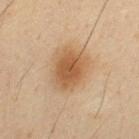The lesion was photographed on a routine skin check and not biopsied; there is no pathology result. A 15 mm crop from a total-body photograph taken for skin-cancer surveillance. A male subject aged approximately 35. The lesion is on the upper back. The total-body-photography lesion software estimated a footprint of about 16 mm², an eccentricity of roughly 0.55, and a symmetry-axis asymmetry near 0.2. The analysis additionally found a mean CIELAB color near L≈50 a*≈17 b*≈33, roughly 10 lightness units darker than nearby skin, and a normalized lesion–skin contrast near 8. It also reported a border-irregularity index near 2/10, a color-variation rating of about 3.5/10, and a peripheral color-asymmetry measure near 1. And it measured an automated nevus-likeness rating near 100 out of 100 and a detector confidence of about 100 out of 100 that the crop contains a lesion. Imaged with cross-polarized lighting.